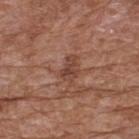Notes:
- workup · no biopsy performed (imaged during a skin exam)
- patient · male, about 75 years old
- diameter · ≈3 mm
- image-analysis metrics · a lesion area of about 4.5 mm² and an outline eccentricity of about 0.8 (0 = round, 1 = elongated); an average lesion color of about L≈43 a*≈22 b*≈27 (CIELAB)
- site · the upper back
- image source · ~15 mm tile from a whole-body skin photo
- lighting · white-light